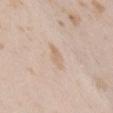Clinical impression:
Captured during whole-body skin photography for melanoma surveillance; the lesion was not biopsied.
Image and clinical context:
Located on the chest. The total-body-photography lesion software estimated border irregularity of about 2.5 on a 0–10 scale and internal color variation of about 0.5 on a 0–10 scale. The software also gave lesion-presence confidence of about 95/100. A 15 mm close-up extracted from a 3D total-body photography capture. About 3.5 mm across. This is a white-light tile. The subject is a female aged 23 to 27.